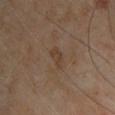| field | value |
|---|---|
| biopsy status | imaged on a skin check; not biopsied |
| diameter | about 3 mm |
| anatomic site | the chest |
| image source | total-body-photography crop, ~15 mm field of view |
| patient | male, roughly 65 years of age |
| automated lesion analysis | a lesion area of about 3.5 mm², an outline eccentricity of about 0.85 (0 = round, 1 = elongated), and two-axis asymmetry of about 0.3 |
| lighting | cross-polarized |